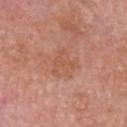workup = total-body-photography surveillance lesion; no biopsy
lesion diameter = ≈4 mm
patient = male, in their mid- to late 70s
anatomic site = the head or neck
imaging modality = ~15 mm tile from a whole-body skin photo